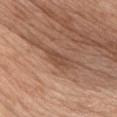notes: total-body-photography surveillance lesion; no biopsy | subject: female, aged 53–57 | automated metrics: a footprint of about 3.5 mm², an eccentricity of roughly 0.9, and a shape-asymmetry score of about 0.3 (0 = symmetric); a mean CIELAB color near L≈48 a*≈21 b*≈30, a lesion–skin lightness drop of about 9, and a normalized border contrast of about 6.5; a border-irregularity rating of about 3/10, a within-lesion color-variation index near 1/10, and radial color variation of about 0; a nevus-likeness score of about 5/100 and a lesion-detection confidence of about 90/100 | image source: total-body-photography crop, ~15 mm field of view | site: the chest | illumination: white-light illumination | lesion diameter: ≈3 mm.